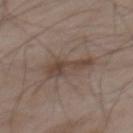biopsy_status: not biopsied; imaged during a skin examination
patient:
  sex: male
  age_approx: 55
image:
  source: total-body photography crop
  field_of_view_mm: 15
site: mid back